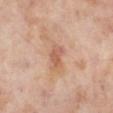Q: Was this lesion biopsied?
A: no biopsy performed (imaged during a skin exam)
Q: How was this image acquired?
A: total-body-photography crop, ~15 mm field of view
Q: Who is the patient?
A: female, in their mid- to late 50s
Q: What did automated image analysis measure?
A: roughly 9 lightness units darker than nearby skin and a lesion-to-skin contrast of about 6 (normalized; higher = more distinct); a color-variation rating of about 1.5/10 and radial color variation of about 0.5; a nevus-likeness score of about 0/100 and a detector confidence of about 100 out of 100 that the crop contains a lesion
Q: What is the anatomic site?
A: the right lower leg